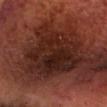Clinical summary:
Automated image analysis of the tile measured an area of roughly 39 mm², a shape eccentricity near 0.65, and a shape-asymmetry score of about 0.35 (0 = symmetric). The analysis additionally found a border-irregularity index near 4.5/10, a within-lesion color-variation index near 5.5/10, and peripheral color asymmetry of about 1.5. And it measured an automated nevus-likeness rating near 0 out of 100 and a detector confidence of about 75 out of 100 that the crop contains a lesion. A 15 mm close-up extracted from a 3D total-body photography capture. From the head or neck. This is a cross-polarized tile. Longest diameter approximately 8.5 mm. The patient is a male roughly 70 years of age.